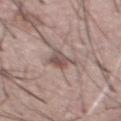Impression:
This lesion was catalogued during total-body skin photography and was not selected for biopsy.
Context:
The recorded lesion diameter is about 3.5 mm. Located on the chest. A 15 mm close-up extracted from a 3D total-body photography capture. A male subject aged approximately 55. Imaged with white-light lighting.